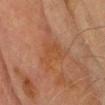Q: Is there a histopathology result?
A: no biopsy performed (imaged during a skin exam)
Q: What did automated image analysis measure?
A: a classifier nevus-likeness of about 0/100 and lesion-presence confidence of about 100/100
Q: How was the tile lit?
A: cross-polarized
Q: Where on the body is the lesion?
A: the head or neck
Q: What is the imaging modality?
A: total-body-photography crop, ~15 mm field of view
Q: Who is the patient?
A: male, about 70 years old